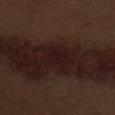workup: no biopsy performed (imaged during a skin exam)
site: the abdomen
illumination: white-light
patient: male, aged approximately 70
image source: total-body-photography crop, ~15 mm field of view